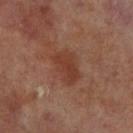Recorded during total-body skin imaging; not selected for excision or biopsy. The patient is a male approximately 70 years of age. A lesion tile, about 15 mm wide, cut from a 3D total-body photograph. Longest diameter approximately 4 mm. An algorithmic analysis of the crop reported a lesion area of about 9 mm², an eccentricity of roughly 0.75, and two-axis asymmetry of about 0.3. The analysis additionally found a mean CIELAB color near L≈37 a*≈23 b*≈28 and a normalized lesion–skin contrast near 6.5. The software also gave border irregularity of about 3 on a 0–10 scale, a color-variation rating of about 2.5/10, and peripheral color asymmetry of about 1. The software also gave an automated nevus-likeness rating near 5 out of 100 and a detector confidence of about 100 out of 100 that the crop contains a lesion. Captured under cross-polarized illumination. The lesion is located on the right lower leg.